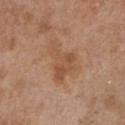Assessment:
This lesion was catalogued during total-body skin photography and was not selected for biopsy.
Background:
Approximately 5.5 mm at its widest. The patient is a female in their mid-70s. A 15 mm close-up tile from a total-body photography series done for melanoma screening. This is a white-light tile. Automated image analysis of the tile measured a symmetry-axis asymmetry near 0.6. The lesion is on the chest.